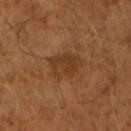<record>
<biopsy_status>not biopsied; imaged during a skin examination</biopsy_status>
<lesion_size>
  <long_diameter_mm_approx>4.0</long_diameter_mm_approx>
</lesion_size>
<automated_metrics>
  <area_mm2_approx>8.5</area_mm2_approx>
  <eccentricity>0.7</eccentricity>
  <vs_skin_darker_L>7.0</vs_skin_darker_L>
  <vs_skin_contrast_norm>6.5</vs_skin_contrast_norm>
  <color_variation_0_10>3.0</color_variation_0_10>
  <nevus_likeness_0_100>0</nevus_likeness_0_100>
  <lesion_detection_confidence_0_100>100</lesion_detection_confidence_0_100>
</automated_metrics>
<lighting>cross-polarized</lighting>
<image>
  <source>total-body photography crop</source>
  <field_of_view_mm>15</field_of_view_mm>
</image>
<patient>
  <sex>male</sex>
  <age_approx>65</age_approx>
</patient>
</record>